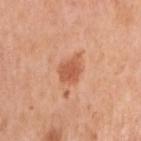The lesion's longest dimension is about 3.5 mm.
The patient is a female aged around 55.
A 15 mm close-up extracted from a 3D total-body photography capture.
The tile uses white-light illumination.
On the right upper arm.